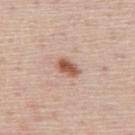Imaged during a routine full-body skin examination; the lesion was not biopsied and no histopathology is available. Captured under white-light illumination. The lesion is on the mid back. A male subject aged around 45. About 3 mm across. Cropped from a whole-body photographic skin survey; the tile spans about 15 mm.